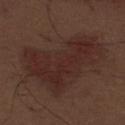Case summary:
– biopsy status · imaged on a skin check; not biopsied
– illumination · white-light illumination
– size · about 9.5 mm
– subject · male, in their 70s
– imaging modality · 15 mm crop, total-body photography
– site · the abdomen
– image-analysis metrics · border irregularity of about 5.5 on a 0–10 scale, a within-lesion color-variation index near 3/10, and a peripheral color-asymmetry measure near 1